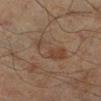Clinical impression: The lesion was tiled from a total-body skin photograph and was not biopsied. Acquisition and patient details: The tile uses cross-polarized illumination. The recorded lesion diameter is about 5 mm. This image is a 15 mm lesion crop taken from a total-body photograph. A male subject about 65 years old. The lesion is on the right lower leg.